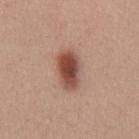{"biopsy_status": "not biopsied; imaged during a skin examination", "image": {"source": "total-body photography crop", "field_of_view_mm": 15}, "lesion_size": {"long_diameter_mm_approx": 4.5}, "patient": {"sex": "male", "age_approx": 30}, "lighting": "white-light", "site": "front of the torso"}